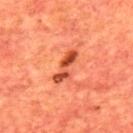Clinical impression:
Part of a total-body skin-imaging series; this lesion was reviewed on a skin check and was not flagged for biopsy.
Acquisition and patient details:
A close-up tile cropped from a whole-body skin photograph, about 15 mm across. From the upper back. The recorded lesion diameter is about 4.5 mm. Captured under cross-polarized illumination. A male subject in their mid-60s.